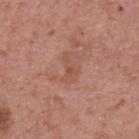notes: no biopsy performed (imaged during a skin exam); patient: male, aged around 70; illumination: white-light; image: 15 mm crop, total-body photography; body site: the upper back; diameter: ≈3.5 mm.